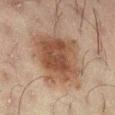biopsy status: imaged on a skin check; not biopsied
image source: 15 mm crop, total-body photography
patient: male, approximately 50 years of age
TBP lesion metrics: border irregularity of about 2.5 on a 0–10 scale and a peripheral color-asymmetry measure near 1.5
body site: the right thigh
lesion diameter: ~6 mm (longest diameter)